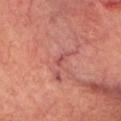image source = ~15 mm tile from a whole-body skin photo
site = the head or neck
patient = female, aged approximately 80